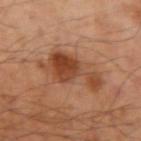biopsy status: imaged on a skin check; not biopsied | diameter: about 6.5 mm | subject: male, about 50 years old | site: the left arm | image source: 15 mm crop, total-body photography.